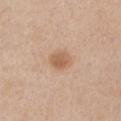Captured during whole-body skin photography for melanoma surveillance; the lesion was not biopsied. Located on the left upper arm. A roughly 15 mm field-of-view crop from a total-body skin photograph. The lesion-visualizer software estimated about 10 CIELAB-L* units darker than the surrounding skin and a normalized border contrast of about 7. A male subject in their 60s. Imaged with white-light lighting.